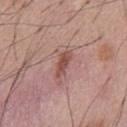Case summary:
– follow-up · imaged on a skin check; not biopsied
– automated lesion analysis · a lesion color around L≈50 a*≈23 b*≈24 in CIELAB, roughly 10 lightness units darker than nearby skin, and a lesion-to-skin contrast of about 7.5 (normalized; higher = more distinct); a border-irregularity rating of about 2.5/10, a color-variation rating of about 2.5/10, and a peripheral color-asymmetry measure near 0.5
– illumination · white-light illumination
– acquisition · ~15 mm crop, total-body skin-cancer survey
– patient · male, aged 53 to 57
– location · the abdomen